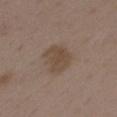Clinical impression: Captured during whole-body skin photography for melanoma surveillance; the lesion was not biopsied.